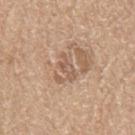{
  "biopsy_status": "not biopsied; imaged during a skin examination",
  "site": "leg",
  "lighting": "white-light",
  "image": {
    "source": "total-body photography crop",
    "field_of_view_mm": 15
  },
  "lesion_size": {
    "long_diameter_mm_approx": 3.0
  },
  "patient": {
    "sex": "female",
    "age_approx": 70
  }
}